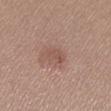<tbp_lesion>
  <biopsy_status>not biopsied; imaged during a skin examination</biopsy_status>
  <automated_metrics>
    <area_mm2_approx>4.0</area_mm2_approx>
    <eccentricity>0.7</eccentricity>
    <shape_asymmetry>0.35</shape_asymmetry>
    <cielab_L>52</cielab_L>
    <cielab_a>21</cielab_a>
    <cielab_b>24</cielab_b>
    <vs_skin_contrast_norm>5.0</vs_skin_contrast_norm>
    <border_irregularity_0_10>3.0</border_irregularity_0_10>
    <color_variation_0_10>2.0</color_variation_0_10>
  </automated_metrics>
  <site>left lower leg</site>
  <image>
    <source>total-body photography crop</source>
    <field_of_view_mm>15</field_of_view_mm>
  </image>
  <patient>
    <sex>female</sex>
    <age_approx>35</age_approx>
  </patient>
  <lesion_size>
    <long_diameter_mm_approx>2.5</long_diameter_mm_approx>
  </lesion_size>
  <lighting>white-light</lighting>
</tbp_lesion>